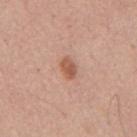– biopsy status · catalogued during a skin exam; not biopsied
– imaging modality · 15 mm crop, total-body photography
– size · ~2.5 mm (longest diameter)
– lighting · white-light
– automated lesion analysis · an area of roughly 4 mm², an outline eccentricity of about 0.75 (0 = round, 1 = elongated), and two-axis asymmetry of about 0.25; border irregularity of about 2 on a 0–10 scale and internal color variation of about 3 on a 0–10 scale; an automated nevus-likeness rating near 90 out of 100 and lesion-presence confidence of about 100/100
– subject · male, aged around 55
– site · the mid back